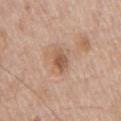Assessment:
Part of a total-body skin-imaging series; this lesion was reviewed on a skin check and was not flagged for biopsy.
Acquisition and patient details:
An algorithmic analysis of the crop reported a lesion color around L≈55 a*≈20 b*≈31 in CIELAB and a normalized border contrast of about 7.5. It also reported a border-irregularity rating of about 1.5/10 and a within-lesion color-variation index near 3/10. The lesion is located on the chest. A 15 mm crop from a total-body photograph taken for skin-cancer surveillance. Longest diameter approximately 3 mm. A male patient approximately 70 years of age.